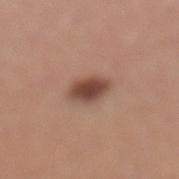notes = total-body-photography surveillance lesion; no biopsy
patient = female, approximately 45 years of age
anatomic site = the leg
size = ~3.5 mm (longest diameter)
acquisition = 15 mm crop, total-body photography
tile lighting = white-light illumination